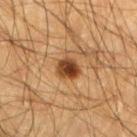| key | value |
|---|---|
| follow-up | no biopsy performed (imaged during a skin exam) |
| automated lesion analysis | a footprint of about 5.5 mm², a shape eccentricity near 0.65, and two-axis asymmetry of about 0.2; a border-irregularity index near 1.5/10 |
| lesion diameter | about 3 mm |
| location | the left lower leg |
| patient | male, aged 48–52 |
| image source | total-body-photography crop, ~15 mm field of view |